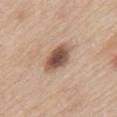notes: catalogued during a skin exam; not biopsied
image: total-body-photography crop, ~15 mm field of view
patient: male, about 60 years old
site: the upper back
tile lighting: white-light illumination